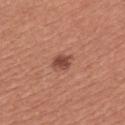Part of a total-body skin-imaging series; this lesion was reviewed on a skin check and was not flagged for biopsy. The lesion is located on the back. Imaged with white-light lighting. A 15 mm close-up tile from a total-body photography series done for melanoma screening. Longest diameter approximately 2.5 mm. A female subject, about 50 years old.